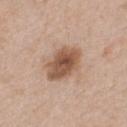The lesion was photographed on a routine skin check and not biopsied; there is no pathology result. A 15 mm close-up extracted from a 3D total-body photography capture. The lesion is located on the chest. The subject is a male aged approximately 50.